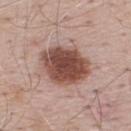Captured during whole-body skin photography for melanoma surveillance; the lesion was not biopsied. The lesion is on the back. Automated tile analysis of the lesion measured a border-irregularity index near 1.5/10, a within-lesion color-variation index near 4.5/10, and peripheral color asymmetry of about 1. The analysis additionally found a classifier nevus-likeness of about 100/100 and a lesion-detection confidence of about 100/100. Captured under white-light illumination. A 15 mm crop from a total-body photograph taken for skin-cancer surveillance. A male subject approximately 70 years of age. About 5.5 mm across.